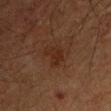* notes — imaged on a skin check; not biopsied
* patient — male, aged 73–77
* tile lighting — cross-polarized illumination
* acquisition — total-body-photography crop, ~15 mm field of view
* site — the left forearm
* diameter — ≈3.5 mm